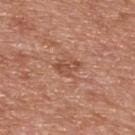Captured during whole-body skin photography for melanoma surveillance; the lesion was not biopsied.
The patient is a male approximately 65 years of age.
From the upper back.
This image is a 15 mm lesion crop taken from a total-body photograph.